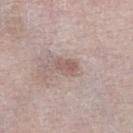Clinical impression: This lesion was catalogued during total-body skin photography and was not selected for biopsy. Image and clinical context: A lesion tile, about 15 mm wide, cut from a 3D total-body photograph. From the right lower leg. The patient is a male aged around 80.